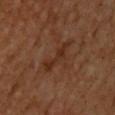The lesion was photographed on a routine skin check and not biopsied; there is no pathology result.
Measured at roughly 4.5 mm in maximum diameter.
A roughly 15 mm field-of-view crop from a total-body skin photograph.
Automated tile analysis of the lesion measured two-axis asymmetry of about 0.4. It also reported a normalized lesion–skin contrast near 6.5. The analysis additionally found a border-irregularity index near 5.5/10, a within-lesion color-variation index near 2/10, and peripheral color asymmetry of about 0.5. It also reported lesion-presence confidence of about 100/100.
A male patient aged around 60.
Captured under cross-polarized illumination.
The lesion is on the back.